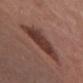Case summary:
- biopsy status · no biopsy performed (imaged during a skin exam)
- patient · male, aged around 50
- diameter · ~5.5 mm (longest diameter)
- acquisition · 15 mm crop, total-body photography
- lighting · white-light illumination
- body site · the chest